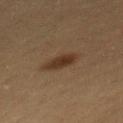biopsy status: catalogued during a skin exam; not biopsied | patient: male, aged approximately 60 | body site: the back | size: about 3.5 mm | acquisition: ~15 mm crop, total-body skin-cancer survey | automated lesion analysis: a footprint of about 5.5 mm² and a shape eccentricity near 0.8; an automated nevus-likeness rating near 100 out of 100 and a lesion-detection confidence of about 100/100.